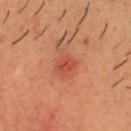  biopsy_status: not biopsied; imaged during a skin examination
  site: head or neck
  image:
    source: total-body photography crop
    field_of_view_mm: 15
  lesion_size:
    long_diameter_mm_approx: 2.5
  lighting: cross-polarized
  patient:
    sex: male
    age_approx: 35